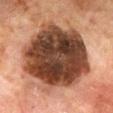Captured during whole-body skin photography for melanoma surveillance; the lesion was not biopsied. A region of skin cropped from a whole-body photographic capture, roughly 15 mm wide. The lesion is located on the chest. An algorithmic analysis of the crop reported a mean CIELAB color near L≈32 a*≈18 b*≈24 and about 19 CIELAB-L* units darker than the surrounding skin. It also reported an automated nevus-likeness rating near 0 out of 100 and a detector confidence of about 100 out of 100 that the crop contains a lesion. Approximately 10.5 mm at its widest. A male subject, in their mid- to late 60s.